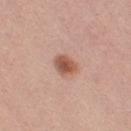The lesion was tiled from a total-body skin photograph and was not biopsied. A roughly 15 mm field-of-view crop from a total-body skin photograph. Located on the left thigh. A female patient, aged 43 to 47. Longest diameter approximately 3 mm. Imaged with white-light lighting.